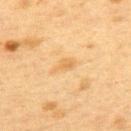  biopsy_status: not biopsied; imaged during a skin examination
  automated_metrics:
    nevus_likeness_0_100: 0
  lesion_size:
    long_diameter_mm_approx: 3.0
  patient:
    sex: female
    age_approx: 40
  image:
    source: total-body photography crop
    field_of_view_mm: 15
  lighting: cross-polarized
  site: upper back